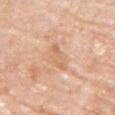| key | value |
|---|---|
| follow-up | no biopsy performed (imaged during a skin exam) |
| location | the chest |
| image source | ~15 mm tile from a whole-body skin photo |
| illumination | white-light illumination |
| patient | male, approximately 75 years of age |